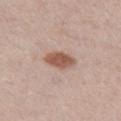No biopsy was performed on this lesion — it was imaged during a full skin examination and was not determined to be concerning.
A 15 mm close-up extracted from a 3D total-body photography capture.
Located on the leg.
A male subject aged 58 to 62.
This is a white-light tile.